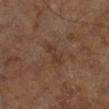Part of a total-body skin-imaging series; this lesion was reviewed on a skin check and was not flagged for biopsy.
The patient is in their mid-60s.
The tile uses cross-polarized illumination.
This image is a 15 mm lesion crop taken from a total-body photograph.
The lesion is located on the right lower leg.
Approximately 3.5 mm at its widest.